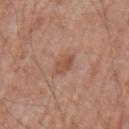Background: The tile uses white-light illumination. The subject is a male approximately 50 years of age. Automated image analysis of the tile measured border irregularity of about 2 on a 0–10 scale and a color-variation rating of about 3.5/10. The software also gave a classifier nevus-likeness of about 15/100 and a lesion-detection confidence of about 100/100. About 2.5 mm across. A region of skin cropped from a whole-body photographic capture, roughly 15 mm wide. The lesion is located on the right upper arm.